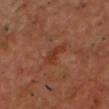Impression:
This lesion was catalogued during total-body skin photography and was not selected for biopsy.
Background:
The lesion is located on the chest. Cropped from a whole-body photographic skin survey; the tile spans about 15 mm. A male subject about 50 years old.